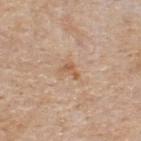Clinical impression: This lesion was catalogued during total-body skin photography and was not selected for biopsy. Background: This image is a 15 mm lesion crop taken from a total-body photograph. A male subject about 55 years old. Automated image analysis of the tile measured border irregularity of about 6 on a 0–10 scale and a color-variation rating of about 0.5/10. It also reported a classifier nevus-likeness of about 0/100. On the upper back.